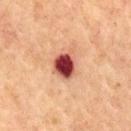Captured during whole-body skin photography for melanoma surveillance; the lesion was not biopsied. A male patient about 65 years old. Cropped from a total-body skin-imaging series; the visible field is about 15 mm. About 3.5 mm across. The total-body-photography lesion software estimated a border-irregularity index near 1.5/10, internal color variation of about 8 on a 0–10 scale, and radial color variation of about 2. The software also gave a classifier nevus-likeness of about 0/100 and lesion-presence confidence of about 100/100. Imaged with cross-polarized lighting. Located on the back.